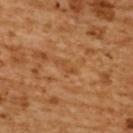biopsy status=catalogued during a skin exam; not biopsied
image=~15 mm tile from a whole-body skin photo
subject=female, aged around 55
location=the upper back
automated metrics=an automated nevus-likeness rating near 0 out of 100 and lesion-presence confidence of about 90/100